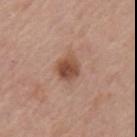Captured during whole-body skin photography for melanoma surveillance; the lesion was not biopsied. A 15 mm close-up tile from a total-body photography series done for melanoma screening. The lesion is on the left upper arm. A female patient aged 63–67. About 3.5 mm across. Imaged with white-light lighting.